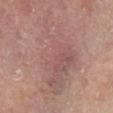biopsy_status: not biopsied; imaged during a skin examination
patient:
  sex: female
  age_approx: 75
lesion_size:
  long_diameter_mm_approx: 11.5
image:
  source: total-body photography crop
  field_of_view_mm: 15
site: left lower leg
lighting: cross-polarized